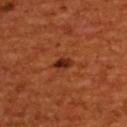Assessment:
Part of a total-body skin-imaging series; this lesion was reviewed on a skin check and was not flagged for biopsy.
Background:
A roughly 15 mm field-of-view crop from a total-body skin photograph. The total-body-photography lesion software estimated an eccentricity of roughly 0.85 and a symmetry-axis asymmetry near 0.3. It also reported a border-irregularity index near 2.5/10 and a peripheral color-asymmetry measure near 1. Measured at roughly 2.5 mm in maximum diameter. A male subject, aged 43–47. From the upper back. This is a cross-polarized tile.